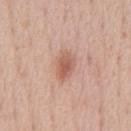biopsy status = imaged on a skin check; not biopsied | TBP lesion metrics = a border-irregularity index near 2/10 and a peripheral color-asymmetry measure near 1.5; a classifier nevus-likeness of about 75/100 | illumination = white-light illumination | patient = male, approximately 55 years of age | image = 15 mm crop, total-body photography | size = ≈4 mm | anatomic site = the chest.